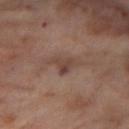Captured during whole-body skin photography for melanoma surveillance; the lesion was not biopsied. The patient is a female in their mid-50s. This is a cross-polarized tile. A 15 mm crop from a total-body photograph taken for skin-cancer surveillance. Longest diameter approximately 3.5 mm. Located on the right thigh.